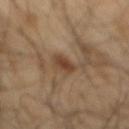Impression:
Recorded during total-body skin imaging; not selected for excision or biopsy.
Clinical summary:
A male subject about 65 years old. Captured under cross-polarized illumination. The lesion is on the mid back. A 15 mm crop from a total-body photograph taken for skin-cancer surveillance.